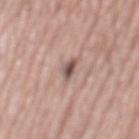biopsy status = total-body-photography surveillance lesion; no biopsy | automated metrics = a footprint of about 3 mm², an eccentricity of roughly 0.95, and a symmetry-axis asymmetry near 0.5 | size = ≈3.5 mm | lighting = white-light illumination | subject = male, aged around 75 | anatomic site = the mid back | image source = 15 mm crop, total-body photography.